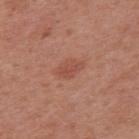{"biopsy_status": "not biopsied; imaged during a skin examination", "patient": {"sex": "female", "age_approx": 40}, "image": {"source": "total-body photography crop", "field_of_view_mm": 15}, "site": "upper back"}